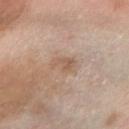Notes:
• follow-up — total-body-photography surveillance lesion; no biopsy
• lesion diameter — about 3 mm
• image — ~15 mm crop, total-body skin-cancer survey
• automated metrics — a lesion color around L≈58 a*≈17 b*≈29 in CIELAB; internal color variation of about 2.5 on a 0–10 scale and a peripheral color-asymmetry measure near 1; a classifier nevus-likeness of about 0/100 and a lesion-detection confidence of about 100/100
• lighting — cross-polarized
• subject — male, about 55 years old
• location — the right forearm